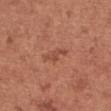Recorded during total-body skin imaging; not selected for excision or biopsy. From the chest. The tile uses white-light illumination. A female subject aged 48 to 52. A 15 mm close-up extracted from a 3D total-body photography capture. The lesion's longest dimension is about 3.5 mm.